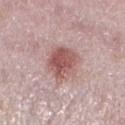Clinical impression:
Part of a total-body skin-imaging series; this lesion was reviewed on a skin check and was not flagged for biopsy.
Context:
The total-body-photography lesion software estimated a lesion color around L≈55 a*≈23 b*≈22 in CIELAB, roughly 12 lightness units darker than nearby skin, and a lesion-to-skin contrast of about 8.5 (normalized; higher = more distinct). The analysis additionally found a border-irregularity index near 2/10, a within-lesion color-variation index near 4.5/10, and a peripheral color-asymmetry measure near 1.5. The lesion is on the left lower leg. A female patient, aged 38 to 42. Cropped from a total-body skin-imaging series; the visible field is about 15 mm. Longest diameter approximately 4.5 mm. The tile uses white-light illumination.